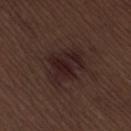Assessment: No biopsy was performed on this lesion — it was imaged during a full skin examination and was not determined to be concerning. Acquisition and patient details: Captured under white-light illumination. A 15 mm close-up extracted from a 3D total-body photography capture. The lesion is located on the lower back. Approximately 5 mm at its widest. A male subject, in their 70s.